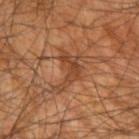notes: total-body-photography surveillance lesion; no biopsy
patient: male, aged around 60
anatomic site: the right arm
diameter: about 5 mm
image: ~15 mm tile from a whole-body skin photo
illumination: cross-polarized